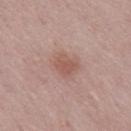Imaged during a routine full-body skin examination; the lesion was not biopsied and no histopathology is available. A 15 mm close-up tile from a total-body photography series done for melanoma screening. The lesion is located on the mid back. This is a white-light tile. An algorithmic analysis of the crop reported a mean CIELAB color near L≈54 a*≈21 b*≈25, a lesion–skin lightness drop of about 8, and a normalized border contrast of about 6. It also reported a nevus-likeness score of about 20/100 and lesion-presence confidence of about 100/100. A male subject, aged approximately 50. The recorded lesion diameter is about 2.5 mm.